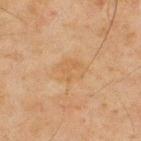{"biopsy_status": "not biopsied; imaged during a skin examination", "patient": {"sex": "male", "age_approx": 45}, "site": "back", "image": {"source": "total-body photography crop", "field_of_view_mm": 15}}